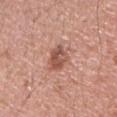Findings:
– biopsy status — catalogued during a skin exam; not biopsied
– location — the left upper arm
– automated lesion analysis — a lesion area of about 6.5 mm² and an eccentricity of roughly 0.5; a border-irregularity index near 2/10 and radial color variation of about 1.5
– illumination — white-light
– size — about 3 mm
– imaging modality — ~15 mm tile from a whole-body skin photo
– subject — male, about 40 years old